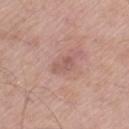Recorded during total-body skin imaging; not selected for excision or biopsy. The lesion's longest dimension is about 3 mm. From the left thigh. An algorithmic analysis of the crop reported a lesion area of about 3 mm², a shape eccentricity near 0.85, and two-axis asymmetry of about 0.5. The software also gave a classifier nevus-likeness of about 0/100 and a detector confidence of about 100 out of 100 that the crop contains a lesion. A male subject aged 53 to 57. Captured under white-light illumination. A region of skin cropped from a whole-body photographic capture, roughly 15 mm wide.